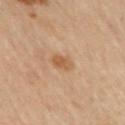Findings:
– notes · total-body-photography surveillance lesion; no biopsy
– image · total-body-photography crop, ~15 mm field of view
– location · the upper back
– lesion diameter · ~2.5 mm (longest diameter)
– patient · female, roughly 60 years of age
– tile lighting · cross-polarized illumination